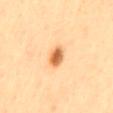This lesion was catalogued during total-body skin photography and was not selected for biopsy. Measured at roughly 3 mm in maximum diameter. From the back. A female subject, aged around 45. Imaged with cross-polarized lighting. A 15 mm close-up tile from a total-body photography series done for melanoma screening. Automated tile analysis of the lesion measured a footprint of about 5 mm², an eccentricity of roughly 0.8, and a symmetry-axis asymmetry near 0.25. And it measured roughly 16 lightness units darker than nearby skin and a lesion-to-skin contrast of about 9.5 (normalized; higher = more distinct). The analysis additionally found a detector confidence of about 100 out of 100 that the crop contains a lesion.